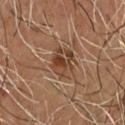Q: Was this lesion biopsied?
A: no biopsy performed (imaged during a skin exam)